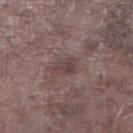| key | value |
|---|---|
| workup | total-body-photography surveillance lesion; no biopsy |
| image source | 15 mm crop, total-body photography |
| subject | male, aged around 75 |
| lighting | white-light |
| lesion size | ≈2.5 mm |
| body site | the leg |